A male subject, in their mid-50s · from the arm · this image is a 15 mm lesion crop taken from a total-body photograph: 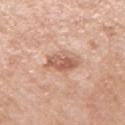The recorded lesion diameter is about 3.5 mm. Biopsy histopathology demonstrated a seborrheic keratosis — a benign skin lesion.